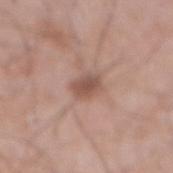biopsy status = imaged on a skin check; not biopsied | patient = male, aged around 70 | acquisition = ~15 mm crop, total-body skin-cancer survey | location = the abdomen.